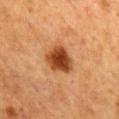The lesion was photographed on a routine skin check and not biopsied; there is no pathology result.
The subject is a female aged approximately 55.
Approximately 4 mm at its widest.
Imaged with cross-polarized lighting.
A lesion tile, about 15 mm wide, cut from a 3D total-body photograph.
The lesion is located on the mid back.
Automated tile analysis of the lesion measured a lesion area of about 10 mm² and an eccentricity of roughly 0.6. It also reported a border-irregularity rating of about 2/10, a within-lesion color-variation index near 4.5/10, and a peripheral color-asymmetry measure near 1. And it measured a classifier nevus-likeness of about 100/100 and a lesion-detection confidence of about 100/100.